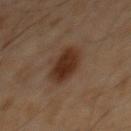Assessment: Recorded during total-body skin imaging; not selected for excision or biopsy. Acquisition and patient details: The lesion's longest dimension is about 5 mm. A male subject, in their 60s. The tile uses cross-polarized illumination. Cropped from a whole-body photographic skin survey; the tile spans about 15 mm. The total-body-photography lesion software estimated a lesion area of about 13 mm², an outline eccentricity of about 0.6 (0 = round, 1 = elongated), and two-axis asymmetry of about 0.1. And it measured an average lesion color of about L≈29 a*≈16 b*≈24 (CIELAB) and a normalized lesion–skin contrast near 10. And it measured a border-irregularity rating of about 1.5/10, a within-lesion color-variation index near 3.5/10, and a peripheral color-asymmetry measure near 1. And it measured an automated nevus-likeness rating near 100 out of 100 and lesion-presence confidence of about 100/100. The lesion is on the chest.